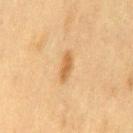notes: imaged on a skin check; not biopsied | patient: female, aged 38–42 | image source: ~15 mm crop, total-body skin-cancer survey | diameter: ≈3.5 mm | tile lighting: cross-polarized | site: the leg | automated metrics: an average lesion color of about L≈54 a*≈18 b*≈38 (CIELAB), about 9 CIELAB-L* units darker than the surrounding skin, and a normalized lesion–skin contrast near 7; a border-irregularity index near 3.5/10, internal color variation of about 1 on a 0–10 scale, and a peripheral color-asymmetry measure near 0.5.